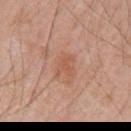biopsy status — catalogued during a skin exam; not biopsied
tile lighting — white-light illumination
lesion diameter — about 3 mm
patient — male, approximately 70 years of age
automated lesion analysis — a footprint of about 3.5 mm², an outline eccentricity of about 0.75 (0 = round, 1 = elongated), and a shape-asymmetry score of about 0.5 (0 = symmetric); a mean CIELAB color near L≈56 a*≈23 b*≈32, a lesion–skin lightness drop of about 7, and a lesion-to-skin contrast of about 5.5 (normalized; higher = more distinct); a border-irregularity index near 5.5/10, a color-variation rating of about 0.5/10, and radial color variation of about 0.5; a classifier nevus-likeness of about 0/100 and a detector confidence of about 100 out of 100 that the crop contains a lesion
body site — the left upper arm
imaging modality — total-body-photography crop, ~15 mm field of view